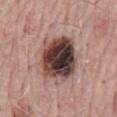Captured during whole-body skin photography for melanoma surveillance; the lesion was not biopsied. This is a white-light tile. The lesion is on the back. The patient is a male aged approximately 70. A 15 mm close-up tile from a total-body photography series done for melanoma screening. The lesion's longest dimension is about 5.5 mm.